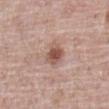Clinical impression: The lesion was photographed on a routine skin check and not biopsied; there is no pathology result. Image and clinical context: The lesion is on the abdomen. A lesion tile, about 15 mm wide, cut from a 3D total-body photograph. A male patient, aged 68 to 72.